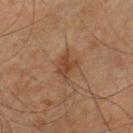This lesion was catalogued during total-body skin photography and was not selected for biopsy.
Measured at roughly 3 mm in maximum diameter.
A male patient, aged around 65.
Captured under cross-polarized illumination.
The lesion is located on the arm.
Cropped from a total-body skin-imaging series; the visible field is about 15 mm.